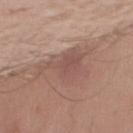Assessment:
No biopsy was performed on this lesion — it was imaged during a full skin examination and was not determined to be concerning.
Background:
On the left upper arm. A male subject aged approximately 45. A region of skin cropped from a whole-body photographic capture, roughly 15 mm wide.